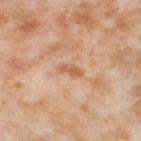Imaged during a routine full-body skin examination; the lesion was not biopsied and no histopathology is available. A female subject, roughly 55 years of age. Captured under cross-polarized illumination. The recorded lesion diameter is about 2.5 mm. The lesion is on the left thigh. A roughly 15 mm field-of-view crop from a total-body skin photograph.